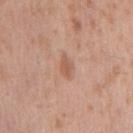Imaged during a routine full-body skin examination; the lesion was not biopsied and no histopathology is available.
Automated tile analysis of the lesion measured a lesion area of about 3 mm², a shape eccentricity near 0.85, and a shape-asymmetry score of about 0.35 (0 = symmetric). The analysis additionally found a mean CIELAB color near L≈57 a*≈22 b*≈31, roughly 8 lightness units darker than nearby skin, and a normalized lesion–skin contrast near 6. And it measured a classifier nevus-likeness of about 5/100.
Longest diameter approximately 2.5 mm.
A male subject, in their mid-40s.
A region of skin cropped from a whole-body photographic capture, roughly 15 mm wide.
On the chest.
Captured under white-light illumination.